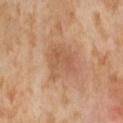The patient is a female aged 53 to 57. The lesion is located on the abdomen. A lesion tile, about 15 mm wide, cut from a 3D total-body photograph.